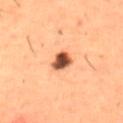{
  "biopsy_status": "not biopsied; imaged during a skin examination",
  "patient": {
    "sex": "male",
    "age_approx": 50
  },
  "image": {
    "source": "total-body photography crop",
    "field_of_view_mm": 15
  },
  "automated_metrics": {
    "eccentricity": 0.3,
    "shape_asymmetry": 0.2,
    "border_irregularity_0_10": 2.0,
    "color_variation_0_10": 6.0,
    "peripheral_color_asymmetry": 1.5
  },
  "site": "abdomen",
  "lesion_size": {
    "long_diameter_mm_approx": 2.5
  }
}